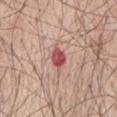{
  "site": "mid back",
  "image": {
    "source": "total-body photography crop",
    "field_of_view_mm": 15
  },
  "lesion_size": {
    "long_diameter_mm_approx": 3.0
  },
  "automated_metrics": {
    "peripheral_color_asymmetry": 2.0,
    "nevus_likeness_0_100": 0,
    "lesion_detection_confidence_0_100": 100
  },
  "lighting": "white-light",
  "patient": {
    "sex": "male",
    "age_approx": 70
  }
}